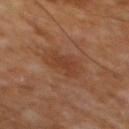notes — no biopsy performed (imaged during a skin exam)
patient — male, approximately 65 years of age
acquisition — total-body-photography crop, ~15 mm field of view
anatomic site — the mid back
tile lighting — cross-polarized
diameter — ≈3.5 mm